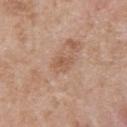Q: Was this lesion biopsied?
A: imaged on a skin check; not biopsied
Q: Lesion location?
A: the chest
Q: What is the imaging modality?
A: total-body-photography crop, ~15 mm field of view
Q: Illumination type?
A: white-light
Q: Who is the patient?
A: male, about 50 years old
Q: Lesion size?
A: ≈2.5 mm